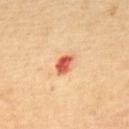Image and clinical context: A 15 mm crop from a total-body photograph taken for skin-cancer surveillance. A male subject aged 63 to 67.